The lesion-visualizer software estimated a nevus-likeness score of about 75/100 · a male subject, aged 78–82 · from the right thigh · a 15 mm close-up tile from a total-body photography series done for melanoma screening · longest diameter approximately 16.5 mm · imaged with white-light lighting — 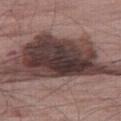Biopsy histopathology demonstrated a melanoma in situ — a malignant skin lesion.